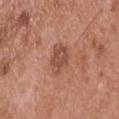Captured during whole-body skin photography for melanoma surveillance; the lesion was not biopsied.
Measured at roughly 3.5 mm in maximum diameter.
An algorithmic analysis of the crop reported an area of roughly 7 mm², an outline eccentricity of about 0.7 (0 = round, 1 = elongated), and a symmetry-axis asymmetry near 0.3. And it measured a lesion–skin lightness drop of about 10 and a normalized lesion–skin contrast near 7. And it measured a classifier nevus-likeness of about 35/100 and a detector confidence of about 100 out of 100 that the crop contains a lesion.
A male patient, aged around 55.
Captured under white-light illumination.
A close-up tile cropped from a whole-body skin photograph, about 15 mm across.
The lesion is on the upper back.